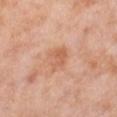workup — catalogued during a skin exam; not biopsied
patient — female, in their mid-50s
acquisition — 15 mm crop, total-body photography
lesion size — ~2.5 mm (longest diameter)
lighting — cross-polarized
body site — the right lower leg